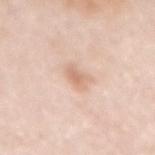This lesion was catalogued during total-body skin photography and was not selected for biopsy. The lesion is on the mid back. An algorithmic analysis of the crop reported an average lesion color of about L≈70 a*≈19 b*≈30 (CIELAB) and a lesion–skin lightness drop of about 9. The software also gave border irregularity of about 3 on a 0–10 scale, a color-variation rating of about 2.5/10, and a peripheral color-asymmetry measure near 0.5. The software also gave a classifier nevus-likeness of about 30/100 and a lesion-detection confidence of about 100/100. A region of skin cropped from a whole-body photographic capture, roughly 15 mm wide. The tile uses white-light illumination. A female subject roughly 50 years of age. Measured at roughly 3 mm in maximum diameter.